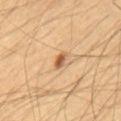The total-body-photography lesion software estimated a footprint of about 2.5 mm², an eccentricity of roughly 0.7, and a symmetry-axis asymmetry near 0.3. And it measured a lesion color around L≈57 a*≈21 b*≈38 in CIELAB and a normalized lesion–skin contrast near 8.5. The analysis additionally found a nevus-likeness score of about 90/100 and a lesion-detection confidence of about 100/100. Longest diameter approximately 2 mm. This is a cross-polarized tile. The subject is a male aged 53 to 57. A lesion tile, about 15 mm wide, cut from a 3D total-body photograph. Located on the mid back.